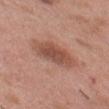The lesion was photographed on a routine skin check and not biopsied; there is no pathology result. Imaged with white-light lighting. The subject is a male roughly 35 years of age. Measured at roughly 6 mm in maximum diameter. A 15 mm close-up extracted from a 3D total-body photography capture. On the head or neck. Automated tile analysis of the lesion measured a lesion area of about 14 mm², a shape eccentricity near 0.85, and a symmetry-axis asymmetry near 0.15. The software also gave a border-irregularity rating of about 2.5/10 and internal color variation of about 3.5 on a 0–10 scale.